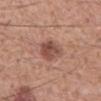Impression:
Part of a total-body skin-imaging series; this lesion was reviewed on a skin check and was not flagged for biopsy.
Background:
Measured at roughly 4 mm in maximum diameter. An algorithmic analysis of the crop reported border irregularity of about 2.5 on a 0–10 scale, a color-variation rating of about 4/10, and radial color variation of about 1.5. The subject is a male aged 53–57. Imaged with white-light lighting. Cropped from a whole-body photographic skin survey; the tile spans about 15 mm. The lesion is on the mid back.